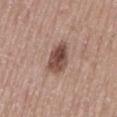| field | value |
|---|---|
| workup | imaged on a skin check; not biopsied |
| tile lighting | white-light |
| image source | ~15 mm crop, total-body skin-cancer survey |
| lesion size | ~4 mm (longest diameter) |
| patient | male, approximately 55 years of age |